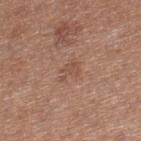Imaged during a routine full-body skin examination; the lesion was not biopsied and no histopathology is available.
A close-up tile cropped from a whole-body skin photograph, about 15 mm across.
The lesion is located on the leg.
Captured under white-light illumination.
An algorithmic analysis of the crop reported a mean CIELAB color near L≈50 a*≈20 b*≈28, a lesion–skin lightness drop of about 6, and a normalized lesion–skin contrast near 5. And it measured a classifier nevus-likeness of about 0/100 and lesion-presence confidence of about 100/100.
The subject is a female aged 38 to 42.
Measured at roughly 3 mm in maximum diameter.